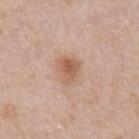Background: Captured under white-light illumination. The total-body-photography lesion software estimated a lesion area of about 6.5 mm² and a symmetry-axis asymmetry near 0.25. And it measured an average lesion color of about L≈59 a*≈20 b*≈31 (CIELAB), about 10 CIELAB-L* units darker than the surrounding skin, and a normalized lesion–skin contrast near 7. The analysis additionally found a nevus-likeness score of about 90/100 and lesion-presence confidence of about 100/100. The lesion's longest dimension is about 3 mm. The lesion is located on the front of the torso. A 15 mm crop from a total-body photograph taken for skin-cancer surveillance. A male subject, roughly 60 years of age.